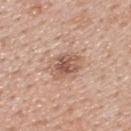Case summary:
- workup · catalogued during a skin exam; not biopsied
- subject · male, aged 38–42
- image source · total-body-photography crop, ~15 mm field of view
- image-analysis metrics · a footprint of about 11 mm², a shape eccentricity near 0.7, and a shape-asymmetry score of about 0.15 (0 = symmetric); a lesion-detection confidence of about 100/100
- body site · the upper back